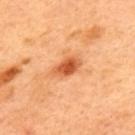Recorded during total-body skin imaging; not selected for excision or biopsy.
A roughly 15 mm field-of-view crop from a total-body skin photograph.
Captured under cross-polarized illumination.
The patient is a male aged approximately 50.
The lesion is on the upper back.
The recorded lesion diameter is about 3.5 mm.